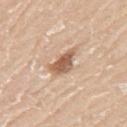{
  "biopsy_status": "not biopsied; imaged during a skin examination",
  "lesion_size": {
    "long_diameter_mm_approx": 4.0
  },
  "image": {
    "source": "total-body photography crop",
    "field_of_view_mm": 15
  },
  "lighting": "white-light",
  "patient": {
    "sex": "male",
    "age_approx": 60
  },
  "automated_metrics": {
    "cielab_L": 59,
    "cielab_a": 20,
    "cielab_b": 32,
    "vs_skin_darker_L": 14.0,
    "peripheral_color_asymmetry": 1.0
  },
  "site": "arm"
}